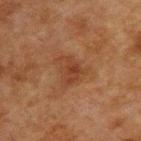| feature | finding |
|---|---|
| notes | imaged on a skin check; not biopsied |
| acquisition | ~15 mm tile from a whole-body skin photo |
| patient | male, aged 58 to 62 |
| anatomic site | the back |
| lesion diameter | ~3.5 mm (longest diameter) |